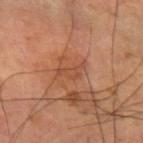Notes:
• notes: no biopsy performed (imaged during a skin exam)
• automated metrics: a lesion area of about 4 mm², an eccentricity of roughly 0.85, and two-axis asymmetry of about 0.5; a lesion color around L≈48 a*≈25 b*≈33 in CIELAB and about 7 CIELAB-L* units darker than the surrounding skin; border irregularity of about 5.5 on a 0–10 scale and internal color variation of about 1.5 on a 0–10 scale; a classifier nevus-likeness of about 0/100
• subject: male, approximately 60 years of age
• tile lighting: cross-polarized
• site: the left forearm
• image source: 15 mm crop, total-body photography